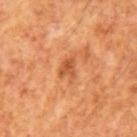{
  "biopsy_status": "not biopsied; imaged during a skin examination",
  "lighting": "cross-polarized",
  "lesion_size": {
    "long_diameter_mm_approx": 2.5
  },
  "image": {
    "source": "total-body photography crop",
    "field_of_view_mm": 15
  },
  "patient": {
    "sex": "male",
    "age_approx": 65
  }
}